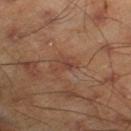size = ≈2.5 mm | tile lighting = cross-polarized | imaging modality = 15 mm crop, total-body photography | TBP lesion metrics = a lesion color around L≈41 a*≈21 b*≈27 in CIELAB and about 6 CIELAB-L* units darker than the surrounding skin; a within-lesion color-variation index near 0.5/10 and radial color variation of about 0; an automated nevus-likeness rating near 0 out of 100 and a lesion-detection confidence of about 100/100 | anatomic site = the right lower leg | subject = male, aged 43 to 47.